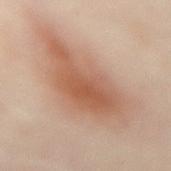biopsy_status: not biopsied; imaged during a skin examination
lighting: cross-polarized
patient:
  sex: female
  age_approx: 50
lesion_size:
  long_diameter_mm_approx: 11.0
site: abdomen
image:
  source: total-body photography crop
  field_of_view_mm: 15